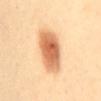| feature | finding |
|---|---|
| biopsy status | total-body-photography surveillance lesion; no biopsy |